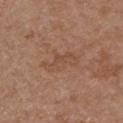Findings:
– follow-up · no biopsy performed (imaged during a skin exam)
– imaging modality · total-body-photography crop, ~15 mm field of view
– diameter · ~5 mm (longest diameter)
– location · the chest
– automated lesion analysis · an area of roughly 8 mm², an eccentricity of roughly 0.9, and a shape-asymmetry score of about 0.35 (0 = symmetric); a border-irregularity rating of about 5.5/10 and radial color variation of about 1
– subject · male, aged 53–57